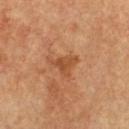{
  "biopsy_status": "not biopsied; imaged during a skin examination",
  "patient": {
    "sex": "male",
    "age_approx": 65
  },
  "image": {
    "source": "total-body photography crop",
    "field_of_view_mm": 15
  },
  "lighting": "cross-polarized",
  "lesion_size": {
    "long_diameter_mm_approx": 3.5
  },
  "site": "chest"
}